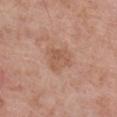workup = total-body-photography surveillance lesion; no biopsy
illumination = white-light
lesion size = ~3.5 mm (longest diameter)
automated metrics = an average lesion color of about L≈56 a*≈21 b*≈30 (CIELAB), about 7 CIELAB-L* units darker than the surrounding skin, and a normalized lesion–skin contrast near 5.5
patient = male, approximately 80 years of age
imaging modality = ~15 mm crop, total-body skin-cancer survey
site = the right lower leg